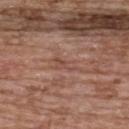Q: How was this image acquired?
A: ~15 mm crop, total-body skin-cancer survey
Q: What did automated image analysis measure?
A: an area of roughly 3.5 mm² and an eccentricity of roughly 0.85; a color-variation rating of about 2.5/10 and a peripheral color-asymmetry measure near 1; an automated nevus-likeness rating near 0 out of 100
Q: Where on the body is the lesion?
A: the upper back
Q: Lesion size?
A: about 3 mm
Q: Patient demographics?
A: female, aged 63 to 67
Q: Illumination type?
A: white-light illumination